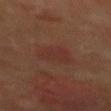Recorded during total-body skin imaging; not selected for excision or biopsy. The lesion is on the chest. This is a cross-polarized tile. The total-body-photography lesion software estimated a lesion area of about 10 mm². The analysis additionally found a mean CIELAB color near L≈29 a*≈20 b*≈21, roughly 4 lightness units darker than nearby skin, and a normalized border contrast of about 4.5. About 4 mm across. The patient is a male in their 60s. A close-up tile cropped from a whole-body skin photograph, about 15 mm across.